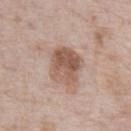Imaged during a routine full-body skin examination; the lesion was not biopsied and no histopathology is available. The lesion's longest dimension is about 4.5 mm. On the abdomen. This image is a 15 mm lesion crop taken from a total-body photograph. The subject is a male aged 68 to 72.